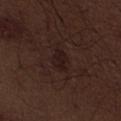Notes:
• notes: no biopsy performed (imaged during a skin exam)
• illumination: white-light
• site: the abdomen
• acquisition: ~15 mm tile from a whole-body skin photo
• subject: male, aged around 70
• automated lesion analysis: an area of roughly 4.5 mm² and an outline eccentricity of about 0.7 (0 = round, 1 = elongated); a mean CIELAB color near L≈17 a*≈16 b*≈15, roughly 5 lightness units darker than nearby skin, and a normalized lesion–skin contrast near 7.5; a border-irregularity rating of about 2.5/10, a color-variation rating of about 1.5/10, and radial color variation of about 0.5
• size: ≈3 mm